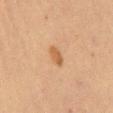No biopsy was performed on this lesion — it was imaged during a full skin examination and was not determined to be concerning.
Imaged with cross-polarized lighting.
Cropped from a whole-body photographic skin survey; the tile spans about 15 mm.
Located on the back.
The recorded lesion diameter is about 3 mm.
A male subject, approximately 60 years of age.
Automated image analysis of the tile measured a shape eccentricity near 0.9 and two-axis asymmetry of about 0.35. The software also gave border irregularity of about 3.5 on a 0–10 scale, internal color variation of about 1 on a 0–10 scale, and a peripheral color-asymmetry measure near 0.5. The analysis additionally found a classifier nevus-likeness of about 85/100 and a detector confidence of about 100 out of 100 that the crop contains a lesion.